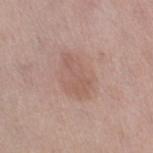This lesion was catalogued during total-body skin photography and was not selected for biopsy. A 15 mm close-up tile from a total-body photography series done for melanoma screening. A female patient, in their 50s. The lesion is located on the leg.